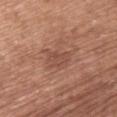<tbp_lesion>
<biopsy_status>not biopsied; imaged during a skin examination</biopsy_status>
<lighting>white-light</lighting>
<lesion_size>
  <long_diameter_mm_approx>3.0</long_diameter_mm_approx>
</lesion_size>
<site>upper back</site>
<patient>
  <sex>female</sex>
  <age_approx>60</age_approx>
</patient>
<image>
  <source>total-body photography crop</source>
  <field_of_view_mm>15</field_of_view_mm>
</image>
<automated_metrics>
  <area_mm2_approx>4.5</area_mm2_approx>
  <eccentricity>0.75</eccentricity>
  <shape_asymmetry>0.45</shape_asymmetry>
  <border_irregularity_0_10>4.5</border_irregularity_0_10>
  <color_variation_0_10>2.0</color_variation_0_10>
  <peripheral_color_asymmetry>0.5</peripheral_color_asymmetry>
  <nevus_likeness_0_100>0</nevus_likeness_0_100>
  <lesion_detection_confidence_0_100>100</lesion_detection_confidence_0_100>
</automated_metrics>
</tbp_lesion>